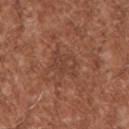notes: total-body-photography surveillance lesion; no biopsy
size: about 4 mm
image-analysis metrics: a mean CIELAB color near L≈42 a*≈22 b*≈28, about 5 CIELAB-L* units darker than the surrounding skin, and a normalized border contrast of about 4.5; a nevus-likeness score of about 0/100 and a lesion-detection confidence of about 95/100
subject: male, aged 43–47
image source: total-body-photography crop, ~15 mm field of view
site: the upper back
illumination: white-light illumination